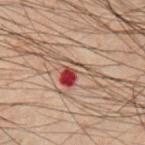notes — catalogued during a skin exam; not biopsied
subject — male, aged 38–42
lighting — cross-polarized illumination
TBP lesion metrics — a mean CIELAB color near L≈39 a*≈21 b*≈23, roughly 10 lightness units darker than nearby skin, and a normalized border contrast of about 9
anatomic site — the left forearm
image source — 15 mm crop, total-body photography
size — ≈4 mm